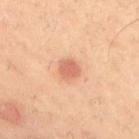biopsy status = total-body-photography surveillance lesion; no biopsy
image source = ~15 mm tile from a whole-body skin photo
anatomic site = the upper back
size = ~2.5 mm (longest diameter)
patient = male, roughly 50 years of age
illumination = cross-polarized illumination